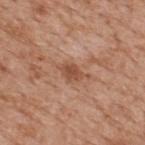Q: Was a biopsy performed?
A: imaged on a skin check; not biopsied
Q: How was the tile lit?
A: white-light illumination
Q: Where on the body is the lesion?
A: the upper back
Q: Patient demographics?
A: male, aged 63–67
Q: Automated lesion metrics?
A: a mean CIELAB color near L≈51 a*≈23 b*≈31 and a normalized border contrast of about 7; border irregularity of about 4 on a 0–10 scale, internal color variation of about 2.5 on a 0–10 scale, and a peripheral color-asymmetry measure near 1; a classifier nevus-likeness of about 0/100 and a detector confidence of about 100 out of 100 that the crop contains a lesion
Q: What is the imaging modality?
A: ~15 mm tile from a whole-body skin photo